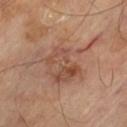Part of a total-body skin-imaging series; this lesion was reviewed on a skin check and was not flagged for biopsy. Cropped from a total-body skin-imaging series; the visible field is about 15 mm. Located on the left thigh. The subject is a male approximately 70 years of age.